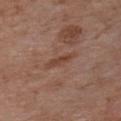No biopsy was performed on this lesion — it was imaged during a full skin examination and was not determined to be concerning.
Automated tile analysis of the lesion measured an area of roughly 4 mm², an eccentricity of roughly 0.95, and a shape-asymmetry score of about 0.3 (0 = symmetric). And it measured a mean CIELAB color near L≈44 a*≈20 b*≈27, about 7 CIELAB-L* units darker than the surrounding skin, and a normalized lesion–skin contrast near 6.5. The analysis additionally found a border-irregularity rating of about 4/10.
The tile uses white-light illumination.
A male subject, in their 60s.
On the chest.
Cropped from a whole-body photographic skin survey; the tile spans about 15 mm.
Longest diameter approximately 4 mm.